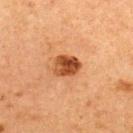Clinical impression:
The lesion was photographed on a routine skin check and not biopsied; there is no pathology result.
Clinical summary:
The lesion's longest dimension is about 3 mm. A female subject, aged around 60. A lesion tile, about 15 mm wide, cut from a 3D total-body photograph. The lesion is located on the upper back. Automated image analysis of the tile measured a mean CIELAB color near L≈40 a*≈24 b*≈34, about 14 CIELAB-L* units darker than the surrounding skin, and a lesion-to-skin contrast of about 11 (normalized; higher = more distinct). It also reported border irregularity of about 1.5 on a 0–10 scale, internal color variation of about 7 on a 0–10 scale, and a peripheral color-asymmetry measure near 3.